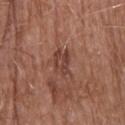biopsy status — catalogued during a skin exam; not biopsied | patient — male, approximately 80 years of age | illumination — white-light | location — the head or neck | imaging modality — 15 mm crop, total-body photography | size — about 4 mm.